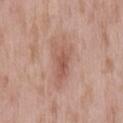Case summary:
• patient: male, aged 53 to 57
• location: the mid back
• diameter: ≈6.5 mm
• image: ~15 mm crop, total-body skin-cancer survey